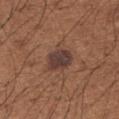Recorded during total-body skin imaging; not selected for excision or biopsy. A male subject, aged around 65. On the left upper arm. An algorithmic analysis of the crop reported a footprint of about 7 mm², an eccentricity of roughly 0.6, and a symmetry-axis asymmetry near 0.2. The software also gave a mean CIELAB color near L≈38 a*≈18 b*≈21 and a normalized lesion–skin contrast near 11. The analysis additionally found a border-irregularity index near 2/10, a color-variation rating of about 3.5/10, and peripheral color asymmetry of about 1. The analysis additionally found an automated nevus-likeness rating near 90 out of 100 and lesion-presence confidence of about 100/100. Measured at roughly 3 mm in maximum diameter. A 15 mm crop from a total-body photograph taken for skin-cancer surveillance.